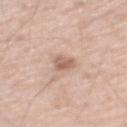The lesion was photographed on a routine skin check and not biopsied; there is no pathology result.
A male subject about 55 years old.
The total-body-photography lesion software estimated border irregularity of about 2.5 on a 0–10 scale and internal color variation of about 3 on a 0–10 scale.
Imaged with white-light lighting.
On the left upper arm.
Approximately 2.5 mm at its widest.
A region of skin cropped from a whole-body photographic capture, roughly 15 mm wide.